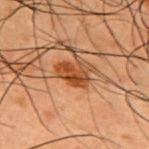Automated tile analysis of the lesion measured internal color variation of about 2.5 on a 0–10 scale and a peripheral color-asymmetry measure near 1. And it measured an automated nevus-likeness rating near 100 out of 100. Located on the upper back. A 15 mm crop from a total-body photograph taken for skin-cancer surveillance. Captured under cross-polarized illumination. A male patient, approximately 50 years of age.